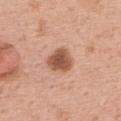| feature | finding |
|---|---|
| follow-up | no biopsy performed (imaged during a skin exam) |
| image | total-body-photography crop, ~15 mm field of view |
| illumination | white-light illumination |
| patient | female, in their 50s |
| body site | the upper back |
| size | ≈3.5 mm |